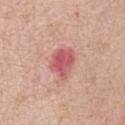Q: Is there a histopathology result?
A: catalogued during a skin exam; not biopsied
Q: What lighting was used for the tile?
A: white-light illumination
Q: How was this image acquired?
A: ~15 mm crop, total-body skin-cancer survey
Q: Lesion size?
A: about 4 mm
Q: Lesion location?
A: the front of the torso
Q: What did automated image analysis measure?
A: an area of roughly 9 mm² and an eccentricity of roughly 0.65; an average lesion color of about L≈57 a*≈32 b*≈23 (CIELAB) and a lesion-to-skin contrast of about 8 (normalized; higher = more distinct); a border-irregularity rating of about 2.5/10 and a color-variation rating of about 5/10; lesion-presence confidence of about 100/100
Q: Patient demographics?
A: female, aged 73 to 77